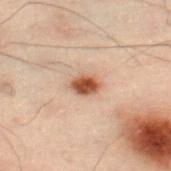Assessment:
This lesion was catalogued during total-body skin photography and was not selected for biopsy.
Clinical summary:
A roughly 15 mm field-of-view crop from a total-body skin photograph. A male patient, in their 50s. Located on the left leg.